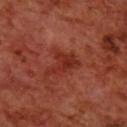| field | value |
|---|---|
| biopsy status | total-body-photography surveillance lesion; no biopsy |
| diameter | ~4.5 mm (longest diameter) |
| image source | 15 mm crop, total-body photography |
| tile lighting | cross-polarized |
| subject | male, aged approximately 70 |
| site | the back |